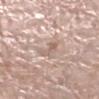Case summary:
* biopsy status: no biopsy performed (imaged during a skin exam)